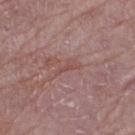  site: left thigh
  lighting: white-light
  image:
    source: total-body photography crop
    field_of_view_mm: 15
  patient:
    sex: female
    age_approx: 65
  lesion_size:
    long_diameter_mm_approx: 2.5